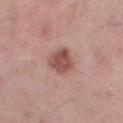Impression:
Imaged during a routine full-body skin examination; the lesion was not biopsied and no histopathology is available.
Context:
The lesion is located on the left thigh. A roughly 15 mm field-of-view crop from a total-body skin photograph. The patient is a male approximately 55 years of age.